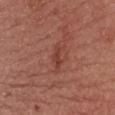No biopsy was performed on this lesion — it was imaged during a full skin examination and was not determined to be concerning.
The total-body-photography lesion software estimated an outline eccentricity of about 0.95 (0 = round, 1 = elongated) and a symmetry-axis asymmetry near 0.45. The analysis additionally found a nevus-likeness score of about 15/100 and a detector confidence of about 100 out of 100 that the crop contains a lesion.
The lesion is located on the chest.
Measured at roughly 2.5 mm in maximum diameter.
Captured under white-light illumination.
A roughly 15 mm field-of-view crop from a total-body skin photograph.
A female patient roughly 65 years of age.